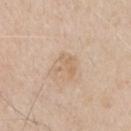No biopsy was performed on this lesion — it was imaged during a full skin examination and was not determined to be concerning. The recorded lesion diameter is about 3 mm. A region of skin cropped from a whole-body photographic capture, roughly 15 mm wide. The subject is a male approximately 65 years of age. The lesion is located on the left upper arm.